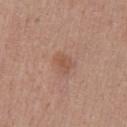biopsy status = imaged on a skin check; not biopsied
imaging modality = ~15 mm crop, total-body skin-cancer survey
lighting = white-light
patient = male, aged 23 to 27
site = the chest